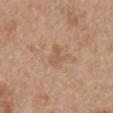Clinical impression: Captured during whole-body skin photography for melanoma surveillance; the lesion was not biopsied. Image and clinical context: From the arm. This image is a 15 mm lesion crop taken from a total-body photograph. A male subject roughly 65 years of age.